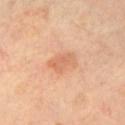workup = imaged on a skin check; not biopsied | subject = male, aged 63–67 | tile lighting = cross-polarized illumination | body site = the left upper arm | imaging modality = ~15 mm tile from a whole-body skin photo.